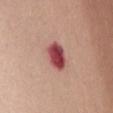patient=female, aged 68 to 72
location=the front of the torso
image source=15 mm crop, total-body photography
lesion size=~4 mm (longest diameter)
tile lighting=white-light